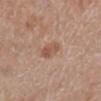Q: Was this lesion biopsied?
A: catalogued during a skin exam; not biopsied
Q: What is the imaging modality?
A: ~15 mm crop, total-body skin-cancer survey
Q: What are the patient's age and sex?
A: female, in their 60s
Q: Lesion location?
A: the leg